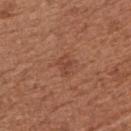imaging modality = ~15 mm tile from a whole-body skin photo
illumination = white-light
site = the right upper arm
patient = female, approximately 65 years of age